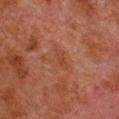The lesion was photographed on a routine skin check and not biopsied; there is no pathology result. A male patient aged 78–82. Automated image analysis of the tile measured a footprint of about 5.5 mm², an outline eccentricity of about 0.75 (0 = round, 1 = elongated), and two-axis asymmetry of about 0.35. The analysis additionally found a border-irregularity rating of about 4/10, a color-variation rating of about 1.5/10, and a peripheral color-asymmetry measure near 0.5. And it measured a lesion-detection confidence of about 100/100. From the right lower leg. This image is a 15 mm lesion crop taken from a total-body photograph. The tile uses cross-polarized illumination.